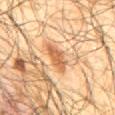Assessment:
The lesion was photographed on a routine skin check and not biopsied; there is no pathology result.
Clinical summary:
The lesion-visualizer software estimated lesion-presence confidence of about 90/100. A 15 mm close-up tile from a total-body photography series done for melanoma screening. The lesion is on the mid back. A male patient, aged 63–67. Imaged with cross-polarized lighting. Approximately 4.5 mm at its widest.